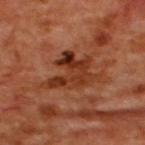biopsy status = catalogued during a skin exam; not biopsied
acquisition = total-body-photography crop, ~15 mm field of view
lighting = cross-polarized
size = ~6.5 mm (longest diameter)
anatomic site = the back
patient = male, about 50 years old